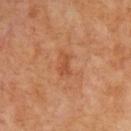The lesion was tiled from a total-body skin photograph and was not biopsied. Measured at roughly 2.5 mm in maximum diameter. Captured under cross-polarized illumination. A male patient in their mid- to late 60s. A roughly 15 mm field-of-view crop from a total-body skin photograph.